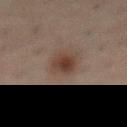Impression: Recorded during total-body skin imaging; not selected for excision or biopsy. Context: A lesion tile, about 15 mm wide, cut from a 3D total-body photograph. The lesion is located on the abdomen. A male subject aged approximately 50. The tile uses cross-polarized illumination. The lesion's longest dimension is about 3.5 mm.